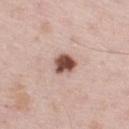biopsy_status: not biopsied; imaged during a skin examination
site: left thigh
image:
  source: total-body photography crop
  field_of_view_mm: 15
lesion_size:
  long_diameter_mm_approx: 3.0
patient:
  sex: male
  age_approx: 60
lighting: white-light
automated_metrics:
  area_mm2_approx: 5.5
  eccentricity: 0.5
  shape_asymmetry: 0.25
  border_irregularity_0_10: 2.0
  color_variation_0_10: 4.5
  peripheral_color_asymmetry: 1.5